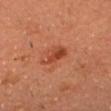The tile uses cross-polarized illumination. The recorded lesion diameter is about 3.5 mm. A roughly 15 mm field-of-view crop from a total-body skin photograph. The patient is a male aged 63–67. The lesion is on the head or neck.